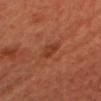Cropped from a whole-body photographic skin survey; the tile spans about 15 mm. The patient is a female aged around 50.